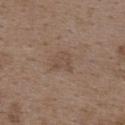  biopsy_status: not biopsied; imaged during a skin examination
  patient:
    sex: female
    age_approx: 35
  site: upper back
  image:
    source: total-body photography crop
    field_of_view_mm: 15
  lighting: white-light